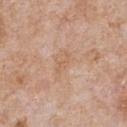– follow-up — imaged on a skin check; not biopsied
– patient — male, aged around 65
– diameter — ~3 mm (longest diameter)
– image — ~15 mm tile from a whole-body skin photo
– body site — the chest